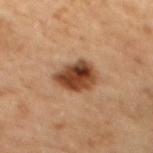Assessment:
Imaged during a routine full-body skin examination; the lesion was not biopsied and no histopathology is available.
Background:
A female subject in their 60s. On the mid back. A region of skin cropped from a whole-body photographic capture, roughly 15 mm wide.